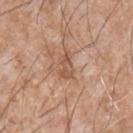Located on the front of the torso.
An algorithmic analysis of the crop reported an area of roughly 4 mm², a shape eccentricity near 0.85, and two-axis asymmetry of about 0.25. It also reported an average lesion color of about L≈54 a*≈21 b*≈30 (CIELAB), a lesion–skin lightness drop of about 9, and a normalized lesion–skin contrast near 6.5.
A lesion tile, about 15 mm wide, cut from a 3D total-body photograph.
A male patient, in their mid-60s.
Measured at roughly 3 mm in maximum diameter.